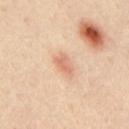notes = total-body-photography surveillance lesion; no biopsy
subject = male, aged 33–37
image source = total-body-photography crop, ~15 mm field of view
site = the upper back